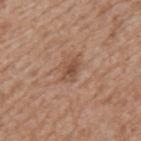– workup: total-body-photography surveillance lesion; no biopsy
– illumination: white-light
– patient: male, in their mid-60s
– diameter: ≈2.5 mm
– site: the mid back
– image source: 15 mm crop, total-body photography
– automated metrics: a lesion area of about 3.5 mm² and an eccentricity of roughly 0.8; a nevus-likeness score of about 20/100